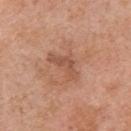| key | value |
|---|---|
| workup | no biopsy performed (imaged during a skin exam) |
| image | ~15 mm tile from a whole-body skin photo |
| subject | male, aged 68–72 |
| anatomic site | the chest |